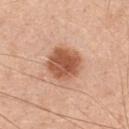  lesion_size:
    long_diameter_mm_approx: 4.5
  lighting: white-light
  image:
    source: total-body photography crop
    field_of_view_mm: 15
  patient:
    sex: male
    age_approx: 50
  site: chest